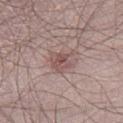  image:
    source: total-body photography crop
    field_of_view_mm: 15
  automated_metrics:
    area_mm2_approx: 6.5
    eccentricity: 0.55
    shape_asymmetry: 0.4
  site: right lower leg
  lesion_size:
    long_diameter_mm_approx: 3.5
  lighting: white-light
  patient:
    sex: male
    age_approx: 25